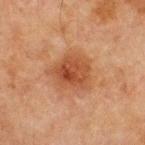No biopsy was performed on this lesion — it was imaged during a full skin examination and was not determined to be concerning. Cropped from a total-body skin-imaging series; the visible field is about 15 mm. Automated tile analysis of the lesion measured an average lesion color of about L≈40 a*≈22 b*≈31 (CIELAB), about 9 CIELAB-L* units darker than the surrounding skin, and a lesion-to-skin contrast of about 7.5 (normalized; higher = more distinct). The software also gave a color-variation rating of about 5/10 and radial color variation of about 1.5. The analysis additionally found an automated nevus-likeness rating near 80 out of 100 and a lesion-detection confidence of about 100/100. A male subject aged 63–67. Measured at roughly 5 mm in maximum diameter. Located on the chest.